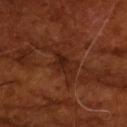follow-up: total-body-photography surveillance lesion; no biopsy
lighting: cross-polarized illumination
patient: male, aged around 65
image source: ~15 mm tile from a whole-body skin photo